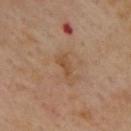Background:
An algorithmic analysis of the crop reported a lesion area of about 3 mm², an eccentricity of roughly 0.9, and a shape-asymmetry score of about 0.3 (0 = symmetric). It also reported a border-irregularity index near 3/10, a within-lesion color-variation index near 0/10, and a peripheral color-asymmetry measure near 0. Cropped from a total-body skin-imaging series; the visible field is about 15 mm. Imaged with cross-polarized lighting. On the upper back. A male patient aged 53–57. About 2.5 mm across.